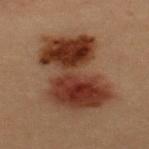biopsy status = imaged on a skin check; not biopsied
subject = female, approximately 30 years of age
site = the upper back
image source = 15 mm crop, total-body photography